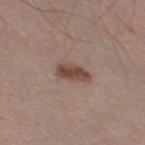The lesion was photographed on a routine skin check and not biopsied; there is no pathology result.
From the left thigh.
Cropped from a whole-body photographic skin survey; the tile spans about 15 mm.
The tile uses white-light illumination.
A male subject, aged 53–57.
About 3.5 mm across.